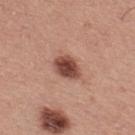{"biopsy_status": "not biopsied; imaged during a skin examination", "patient": {"sex": "male", "age_approx": 40}, "site": "upper back", "lighting": "white-light", "lesion_size": {"long_diameter_mm_approx": 3.5}, "image": {"source": "total-body photography crop", "field_of_view_mm": 15}}